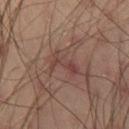{
  "lighting": "cross-polarized",
  "site": "right thigh",
  "image": {
    "source": "total-body photography crop",
    "field_of_view_mm": 15
  },
  "lesion_size": {
    "long_diameter_mm_approx": 3.5
  },
  "patient": {
    "sex": "male",
    "age_approx": 40
  }
}